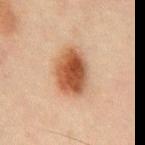Notes:
- follow-up · no biopsy performed (imaged during a skin exam)
- image · total-body-photography crop, ~15 mm field of view
- lesion diameter · ~5 mm (longest diameter)
- subject · male, aged around 45
- illumination · cross-polarized
- anatomic site · the abdomen